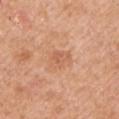The lesion was photographed on a routine skin check and not biopsied; there is no pathology result. The patient is a male roughly 65 years of age. Captured under white-light illumination. The lesion is located on the left upper arm. A 15 mm close-up tile from a total-body photography series done for melanoma screening.